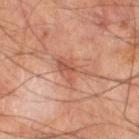<record>
  <biopsy_status>not biopsied; imaged during a skin examination</biopsy_status>
  <site>leg</site>
  <patient>
    <sex>male</sex>
    <age_approx>65</age_approx>
  </patient>
  <lesion_size>
    <long_diameter_mm_approx>4.0</long_diameter_mm_approx>
  </lesion_size>
  <image>
    <source>total-body photography crop</source>
    <field_of_view_mm>15</field_of_view_mm>
  </image>
  <lighting>cross-polarized</lighting>
</record>